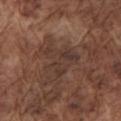No biopsy was performed on this lesion — it was imaged during a full skin examination and was not determined to be concerning. From the left upper arm. A male patient, in their mid-70s. A 15 mm crop from a total-body photograph taken for skin-cancer surveillance.